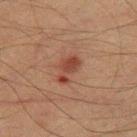| key | value |
|---|---|
| workup | no biopsy performed (imaged during a skin exam) |
| location | the right thigh |
| lesion size | about 3.5 mm |
| image-analysis metrics | a lesion area of about 5 mm², a shape eccentricity near 0.85, and a symmetry-axis asymmetry near 0.45; border irregularity of about 5.5 on a 0–10 scale, a color-variation rating of about 2.5/10, and a peripheral color-asymmetry measure near 1; a classifier nevus-likeness of about 0/100 |
| patient | male, about 50 years old |
| acquisition | ~15 mm crop, total-body skin-cancer survey |
| tile lighting | cross-polarized |